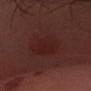The lesion was tiled from a total-body skin photograph and was not biopsied. Located on the head or neck. A male patient aged approximately 40. Imaged with white-light lighting. The recorded lesion diameter is about 4.5 mm. A lesion tile, about 15 mm wide, cut from a 3D total-body photograph.